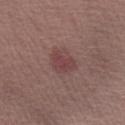<lesion>
  <biopsy_status>not biopsied; imaged during a skin examination</biopsy_status>
  <image>
    <source>total-body photography crop</source>
    <field_of_view_mm>15</field_of_view_mm>
  </image>
  <patient>
    <sex>male</sex>
    <age_approx>30</age_approx>
  </patient>
  <lighting>white-light</lighting>
  <lesion_size>
    <long_diameter_mm_approx>3.5</long_diameter_mm_approx>
  </lesion_size>
</lesion>